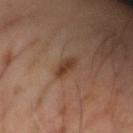No biopsy was performed on this lesion — it was imaged during a full skin examination and was not determined to be concerning. A male subject roughly 65 years of age. The lesion-visualizer software estimated a footprint of about 3.5 mm², an eccentricity of roughly 0.8, and a shape-asymmetry score of about 0.25 (0 = symmetric). It also reported an average lesion color of about L≈37 a*≈18 b*≈28 (CIELAB) and a normalized border contrast of about 8.5. It also reported an automated nevus-likeness rating near 85 out of 100 and a lesion-detection confidence of about 100/100. A 15 mm close-up tile from a total-body photography series done for melanoma screening.